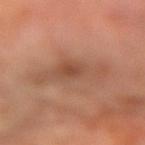follow-up = no biopsy performed (imaged during a skin exam); image source = 15 mm crop, total-body photography; lesion diameter = ~6.5 mm (longest diameter); subject = male, aged 68 to 72; lighting = cross-polarized illumination; anatomic site = the left forearm; automated lesion analysis = an automated nevus-likeness rating near 0 out of 100 and lesion-presence confidence of about 100/100.